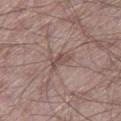This lesion was catalogued during total-body skin photography and was not selected for biopsy. From the right thigh. A male patient aged 63–67. A 15 mm crop from a total-body photograph taken for skin-cancer surveillance.